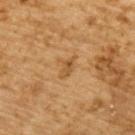follow-up — imaged on a skin check; not biopsied | imaging modality — ~15 mm tile from a whole-body skin photo | lesion size — about 2.5 mm | anatomic site — the upper back | subject — male, aged 83 or older.